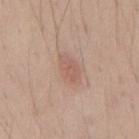{
  "biopsy_status": "not biopsied; imaged during a skin examination",
  "site": "back",
  "image": {
    "source": "total-body photography crop",
    "field_of_view_mm": 15
  },
  "patient": {
    "sex": "male",
    "age_approx": 40
  }
}